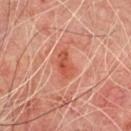Clinical impression: Part of a total-body skin-imaging series; this lesion was reviewed on a skin check and was not flagged for biopsy. Background: Located on the chest. Cropped from a total-body skin-imaging series; the visible field is about 15 mm. Measured at roughly 3.5 mm in maximum diameter. A male patient, approximately 65 years of age.